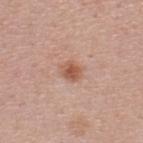Clinical impression:
Captured during whole-body skin photography for melanoma surveillance; the lesion was not biopsied.
Acquisition and patient details:
Automated tile analysis of the lesion measured an average lesion color of about L≈56 a*≈23 b*≈30 (CIELAB), about 10 CIELAB-L* units darker than the surrounding skin, and a normalized border contrast of about 7.5. The analysis additionally found internal color variation of about 3 on a 0–10 scale. It also reported a classifier nevus-likeness of about 90/100 and lesion-presence confidence of about 100/100. From the upper back. A close-up tile cropped from a whole-body skin photograph, about 15 mm across. A male patient aged approximately 45. Measured at roughly 2.5 mm in maximum diameter. This is a white-light tile.